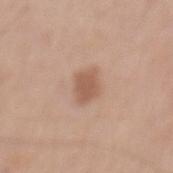Recorded during total-body skin imaging; not selected for excision or biopsy.
About 3 mm across.
The tile uses white-light illumination.
Located on the mid back.
A 15 mm close-up extracted from a 3D total-body photography capture.
The total-body-photography lesion software estimated an area of roughly 5.5 mm² and a symmetry-axis asymmetry near 0.3. The software also gave a mean CIELAB color near L≈56 a*≈20 b*≈29 and a lesion-to-skin contrast of about 7 (normalized; higher = more distinct). The analysis additionally found a nevus-likeness score of about 80/100 and a lesion-detection confidence of about 100/100.
The patient is a male roughly 65 years of age.